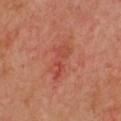{"biopsy_status": "not biopsied; imaged during a skin examination", "site": "chest", "lighting": "cross-polarized", "image": {"source": "total-body photography crop", "field_of_view_mm": 15}, "patient": {"sex": "female", "age_approx": 55}, "lesion_size": {"long_diameter_mm_approx": 5.0}}